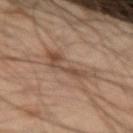Notes:
- follow-up: imaged on a skin check; not biopsied
- size: ≈5.5 mm
- patient: male, approximately 35 years of age
- anatomic site: the arm
- TBP lesion metrics: a footprint of about 9.5 mm², an outline eccentricity of about 0.9 (0 = round, 1 = elongated), and a symmetry-axis asymmetry near 0.55; a detector confidence of about 75 out of 100 that the crop contains a lesion
- acquisition: 15 mm crop, total-body photography